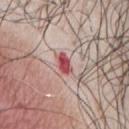The tile uses white-light illumination. A 15 mm close-up extracted from a 3D total-body photography capture. Located on the chest. Automated tile analysis of the lesion measured an average lesion color of about L≈51 a*≈33 b*≈22 (CIELAB), a lesion–skin lightness drop of about 15, and a normalized border contrast of about 10.5. It also reported a border-irregularity rating of about 2.5/10 and radial color variation of about 1.5. And it measured a nevus-likeness score of about 0/100 and a lesion-detection confidence of about 100/100. A male patient in their 70s. Approximately 2.5 mm at its widest.